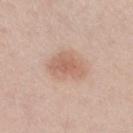The lesion was photographed on a routine skin check and not biopsied; there is no pathology result.
Imaged with white-light lighting.
The subject is a male aged 58–62.
About 4.5 mm across.
A 15 mm crop from a total-body photograph taken for skin-cancer surveillance.
The lesion is on the right thigh.